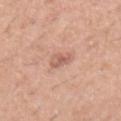Q: Is there a histopathology result?
A: catalogued during a skin exam; not biopsied
Q: What lighting was used for the tile?
A: white-light
Q: What is the imaging modality?
A: total-body-photography crop, ~15 mm field of view
Q: Lesion location?
A: the right forearm
Q: Lesion size?
A: ≈2.5 mm
Q: What are the patient's age and sex?
A: male, roughly 40 years of age